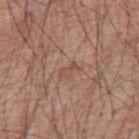{
  "biopsy_status": "not biopsied; imaged during a skin examination",
  "patient": {
    "sex": "male",
    "age_approx": 55
  },
  "site": "mid back",
  "image": {
    "source": "total-body photography crop",
    "field_of_view_mm": 15
  }
}